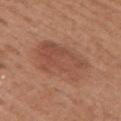| key | value |
|---|---|
| patient | female, roughly 40 years of age |
| illumination | white-light illumination |
| location | the right upper arm |
| image | 15 mm crop, total-body photography |